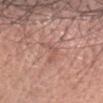  biopsy_status: not biopsied; imaged during a skin examination
  automated_metrics:
    eccentricity: 0.9
    shape_asymmetry: 0.65
    cielab_L: 55
    cielab_a: 23
    cielab_b: 25
    vs_skin_darker_L: 7.0
    border_irregularity_0_10: 7.0
    color_variation_0_10: 0.0
    peripheral_color_asymmetry: 0.0
    lesion_detection_confidence_0_100: 85
  lesion_size:
    long_diameter_mm_approx: 3.5
  image:
    source: total-body photography crop
    field_of_view_mm: 15
  lighting: white-light
  patient:
    sex: female
    age_approx: 65
  site: head or neck